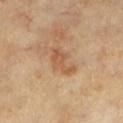Impression: Part of a total-body skin-imaging series; this lesion was reviewed on a skin check and was not flagged for biopsy. Image and clinical context: A female patient, in their 60s. A 15 mm close-up tile from a total-body photography series done for melanoma screening. An algorithmic analysis of the crop reported an outline eccentricity of about 0.8 (0 = round, 1 = elongated) and two-axis asymmetry of about 0.4. It also reported a mean CIELAB color near L≈57 a*≈21 b*≈36, a lesion–skin lightness drop of about 8, and a lesion-to-skin contrast of about 6 (normalized; higher = more distinct). It also reported internal color variation of about 2 on a 0–10 scale and peripheral color asymmetry of about 0.5. The software also gave an automated nevus-likeness rating near 0 out of 100. The lesion is on the leg. Longest diameter approximately 4.5 mm.